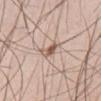Case summary:
– imaging modality — 15 mm crop, total-body photography
– subject — male, roughly 35 years of age
– anatomic site — the lower back